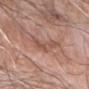No biopsy was performed on this lesion — it was imaged during a full skin examination and was not determined to be concerning.
Cropped from a whole-body photographic skin survey; the tile spans about 15 mm.
The patient is a male aged 58 to 62.
From the right forearm.
The tile uses white-light illumination.
Longest diameter approximately 5.5 mm.